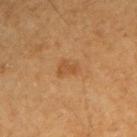  lighting: cross-polarized
  image:
    source: total-body photography crop
    field_of_view_mm: 15
  site: right upper arm
  patient:
    sex: male
    age_approx: 60